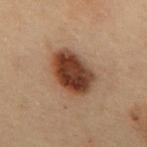<case>
  <site>back</site>
  <automated_metrics>
    <area_mm2_approx>19.0</area_mm2_approx>
    <border_irregularity_0_10>1.5</border_irregularity_0_10>
    <peripheral_color_asymmetry>2.0</peripheral_color_asymmetry>
  </automated_metrics>
  <image>
    <source>total-body photography crop</source>
    <field_of_view_mm>15</field_of_view_mm>
  </image>
  <lesion_size>
    <long_diameter_mm_approx>5.5</long_diameter_mm_approx>
  </lesion_size>
  <lighting>cross-polarized</lighting>
  <patient>
    <sex>male</sex>
    <age_approx>55</age_approx>
  </patient>
</case>